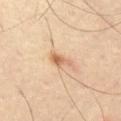follow-up=catalogued during a skin exam; not biopsied
body site=the left thigh
lesion diameter=about 3 mm
lighting=cross-polarized
image source=~15 mm crop, total-body skin-cancer survey
image-analysis metrics=internal color variation of about 1.5 on a 0–10 scale and peripheral color asymmetry of about 0.5; an automated nevus-likeness rating near 75 out of 100 and a lesion-detection confidence of about 100/100